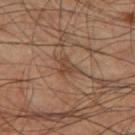Q: What did automated image analysis measure?
A: internal color variation of about 1.5 on a 0–10 scale and peripheral color asymmetry of about 0.5; an automated nevus-likeness rating near 0 out of 100 and lesion-presence confidence of about 70/100
Q: Where on the body is the lesion?
A: the left thigh
Q: What is the lesion's diameter?
A: about 2.5 mm
Q: Illumination type?
A: cross-polarized illumination
Q: Patient demographics?
A: male, aged around 60
Q: What is the imaging modality?
A: total-body-photography crop, ~15 mm field of view